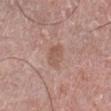Assessment: Imaged during a routine full-body skin examination; the lesion was not biopsied and no histopathology is available. Image and clinical context: A 15 mm close-up extracted from a 3D total-body photography capture. The tile uses white-light illumination. The recorded lesion diameter is about 3 mm. The lesion is located on the leg. The patient is a male about 75 years old.